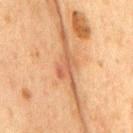Q: Lesion location?
A: the chest
Q: What lighting was used for the tile?
A: cross-polarized
Q: How large is the lesion?
A: ~3.5 mm (longest diameter)
Q: What is the imaging modality?
A: ~15 mm tile from a whole-body skin photo
Q: Patient demographics?
A: male, in their mid-70s